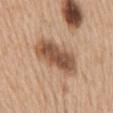follow-up: total-body-photography surveillance lesion; no biopsy
tile lighting: white-light
lesion size: about 7.5 mm
body site: the mid back
imaging modality: ~15 mm tile from a whole-body skin photo
subject: female, approximately 70 years of age